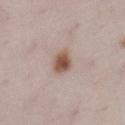The lesion was tiled from a total-body skin photograph and was not biopsied.
A female subject in their 30s.
From the left lower leg.
The recorded lesion diameter is about 2.5 mm.
A 15 mm close-up extracted from a 3D total-body photography capture.